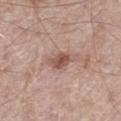- workup: imaged on a skin check; not biopsied
- location: the left thigh
- image source: 15 mm crop, total-body photography
- diameter: about 3 mm
- illumination: white-light
- subject: male, aged approximately 70